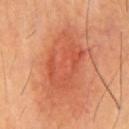Recorded during total-body skin imaging; not selected for excision or biopsy. The total-body-photography lesion software estimated a border-irregularity rating of about 2.5/10, internal color variation of about 4.5 on a 0–10 scale, and a peripheral color-asymmetry measure near 1.5. From the chest. A male subject, in their 60s. This is a cross-polarized tile. About 8.5 mm across. A roughly 15 mm field-of-view crop from a total-body skin photograph.